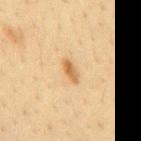Captured during whole-body skin photography for melanoma surveillance; the lesion was not biopsied. This is a cross-polarized tile. The lesion is on the back. An algorithmic analysis of the crop reported a footprint of about 3 mm², an eccentricity of roughly 0.9, and a symmetry-axis asymmetry near 0.2. It also reported an average lesion color of about L≈59 a*≈18 b*≈40 (CIELAB) and a lesion–skin lightness drop of about 11. The patient is a male aged around 35. Measured at roughly 3 mm in maximum diameter. A 15 mm close-up extracted from a 3D total-body photography capture.